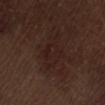Q: Was a biopsy performed?
A: total-body-photography surveillance lesion; no biopsy
Q: What is the anatomic site?
A: the lower back
Q: How was this image acquired?
A: ~15 mm crop, total-body skin-cancer survey
Q: Lesion size?
A: ≈5.5 mm
Q: Who is the patient?
A: male, about 70 years old
Q: How was the tile lit?
A: white-light illumination
Q: What did automated image analysis measure?
A: a footprint of about 8.5 mm² and an outline eccentricity of about 0.9 (0 = round, 1 = elongated); a border-irregularity rating of about 4.5/10, a color-variation rating of about 2.5/10, and radial color variation of about 1; lesion-presence confidence of about 95/100